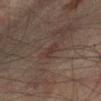notes=catalogued during a skin exam; not biopsied | image=total-body-photography crop, ~15 mm field of view | lesion diameter=about 2.5 mm | location=the leg | subject=male, aged 73–77.